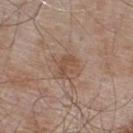<case>
<biopsy_status>not biopsied; imaged during a skin examination</biopsy_status>
<image>
  <source>total-body photography crop</source>
  <field_of_view_mm>15</field_of_view_mm>
</image>
<site>upper back</site>
<lighting>white-light</lighting>
<patient>
  <sex>male</sex>
  <age_approx>55</age_approx>
</patient>
</case>